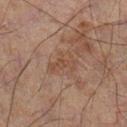workup = imaged on a skin check; not biopsied | imaging modality = ~15 mm crop, total-body skin-cancer survey | body site = the left leg | patient = male, approximately 60 years of age | diameter = ~3.5 mm (longest diameter) | automated metrics = a footprint of about 5.5 mm², a shape eccentricity near 0.8, and a symmetry-axis asymmetry near 0.5; a color-variation rating of about 1.5/10 and peripheral color asymmetry of about 0.5; a classifier nevus-likeness of about 0/100 and lesion-presence confidence of about 100/100 | illumination = cross-polarized.